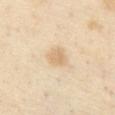| key | value |
|---|---|
| workup | catalogued during a skin exam; not biopsied |
| body site | the abdomen |
| lesion size | about 2.5 mm |
| imaging modality | ~15 mm tile from a whole-body skin photo |
| tile lighting | cross-polarized illumination |
| patient | female, approximately 55 years of age |